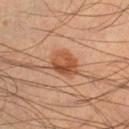follow-up: total-body-photography surveillance lesion; no biopsy
site: the left lower leg
illumination: cross-polarized
acquisition: ~15 mm tile from a whole-body skin photo
subject: male, in their mid- to late 40s